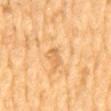  biopsy_status: not biopsied; imaged during a skin examination
  patient:
    sex: male
    age_approx: 85+
  image:
    source: total-body photography crop
    field_of_view_mm: 15
  lesion_size:
    long_diameter_mm_approx: 2.5
  site: back